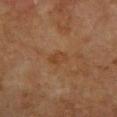Assessment:
This lesion was catalogued during total-body skin photography and was not selected for biopsy.
Context:
The lesion is located on the arm. This image is a 15 mm lesion crop taken from a total-body photograph. Approximately 2.5 mm at its widest. An algorithmic analysis of the crop reported a lesion–skin lightness drop of about 5 and a normalized border contrast of about 5.5. It also reported border irregularity of about 2.5 on a 0–10 scale and internal color variation of about 2 on a 0–10 scale. The software also gave a nevus-likeness score of about 0/100. This is a cross-polarized tile. A female patient, in their 80s.